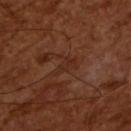Impression: The lesion was tiled from a total-body skin photograph and was not biopsied. Image and clinical context: Measured at roughly 3 mm in maximum diameter. The tile uses cross-polarized illumination. A male subject in their mid- to late 60s. Cropped from a whole-body photographic skin survey; the tile spans about 15 mm. An algorithmic analysis of the crop reported a lesion area of about 4 mm², an outline eccentricity of about 0.85 (0 = round, 1 = elongated), and a symmetry-axis asymmetry near 0.45. It also reported a lesion color around L≈27 a*≈21 b*≈26 in CIELAB and a lesion-to-skin contrast of about 5 (normalized; higher = more distinct).